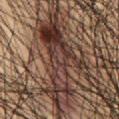The lesion was tiled from a total-body skin photograph and was not biopsied.
The subject is a male approximately 50 years of age.
A region of skin cropped from a whole-body photographic capture, roughly 15 mm wide.
The lesion is on the front of the torso.